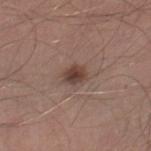Recorded during total-body skin imaging; not selected for excision or biopsy. The lesion is located on the right lower leg. A male subject, aged around 30. Approximately 2.5 mm at its widest. Cropped from a total-body skin-imaging series; the visible field is about 15 mm. Automated tile analysis of the lesion measured a lesion–skin lightness drop of about 11 and a normalized border contrast of about 9.